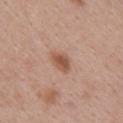workup: no biopsy performed (imaged during a skin exam) | lesion size: ~3.5 mm (longest diameter) | image: ~15 mm crop, total-body skin-cancer survey | image-analysis metrics: an area of roughly 5.5 mm² and an outline eccentricity of about 0.75 (0 = round, 1 = elongated); a classifier nevus-likeness of about 95/100 and a lesion-detection confidence of about 100/100 | anatomic site: the right upper arm | patient: female, aged around 40.